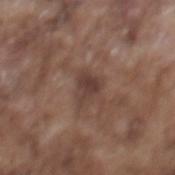A roughly 15 mm field-of-view crop from a total-body skin photograph. The lesion is located on the mid back. The subject is a male in their mid- to late 70s.